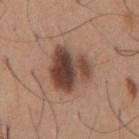Notes:
* biopsy status: imaged on a skin check; not biopsied
* patient: male, roughly 65 years of age
* image source: 15 mm crop, total-body photography
* body site: the chest